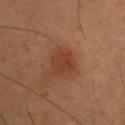follow-up: catalogued during a skin exam; not biopsied
image-analysis metrics: an average lesion color of about L≈31 a*≈20 b*≈27 (CIELAB) and about 6 CIELAB-L* units darker than the surrounding skin
image source: ~15 mm tile from a whole-body skin photo
anatomic site: the right upper arm
patient: male, in their mid-50s